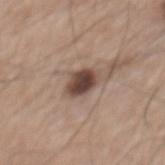workup: total-body-photography surveillance lesion; no biopsy
lighting: white-light
lesion diameter: ~3.5 mm (longest diameter)
location: the mid back
patient: male, in their mid-70s
image: ~15 mm tile from a whole-body skin photo
image-analysis metrics: an average lesion color of about L≈44 a*≈17 b*≈22 (CIELAB), a lesion–skin lightness drop of about 16, and a lesion-to-skin contrast of about 12 (normalized; higher = more distinct); lesion-presence confidence of about 100/100